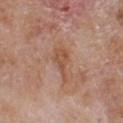Part of a total-body skin-imaging series; this lesion was reviewed on a skin check and was not flagged for biopsy.
A close-up tile cropped from a whole-body skin photograph, about 15 mm across.
Automated image analysis of the tile measured border irregularity of about 6 on a 0–10 scale, internal color variation of about 2.5 on a 0–10 scale, and radial color variation of about 1.
About 4.5 mm across.
The patient is a male in their mid-60s.
The lesion is on the chest.
The tile uses white-light illumination.